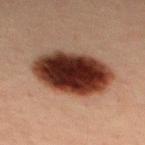Findings:
• workup: catalogued during a skin exam; not biopsied
• lesion diameter: ≈8 mm
• image source: 15 mm crop, total-body photography
• subject: male, approximately 30 years of age
• site: the mid back
• illumination: cross-polarized illumination